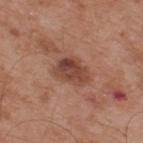{"biopsy_status": "not biopsied; imaged during a skin examination", "patient": {"sex": "male", "age_approx": 55}, "image": {"source": "total-body photography crop", "field_of_view_mm": 15}, "automated_metrics": {"border_irregularity_0_10": 2.5, "color_variation_0_10": 5.5, "nevus_likeness_0_100": 10, "lesion_detection_confidence_0_100": 100}, "site": "upper back", "lesion_size": {"long_diameter_mm_approx": 4.0}}